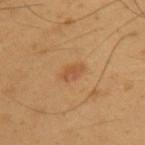{"biopsy_status": "not biopsied; imaged during a skin examination", "site": "upper back", "image": {"source": "total-body photography crop", "field_of_view_mm": 15}, "automated_metrics": {"area_mm2_approx": 3.0, "eccentricity": 0.85, "shape_asymmetry": 0.25, "cielab_L": 50, "cielab_a": 22, "cielab_b": 37, "vs_skin_darker_L": 7.0, "vs_skin_contrast_norm": 5.5, "border_irregularity_0_10": 3.0, "color_variation_0_10": 1.5, "nevus_likeness_0_100": 35, "lesion_detection_confidence_0_100": 100}, "patient": {"sex": "male", "age_approx": 55}, "lesion_size": {"long_diameter_mm_approx": 2.5}, "lighting": "cross-polarized"}